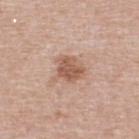size — ~3.5 mm (longest diameter)
imaging modality — ~15 mm crop, total-body skin-cancer survey
automated metrics — an area of roughly 8.5 mm², an outline eccentricity of about 0.45 (0 = round, 1 = elongated), and a shape-asymmetry score of about 0.35 (0 = symmetric); a border-irregularity rating of about 3.5/10, a color-variation rating of about 3.5/10, and a peripheral color-asymmetry measure near 1
patient — female, approximately 40 years of age
lighting — white-light illumination
location — the upper back